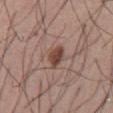Assessment: Recorded during total-body skin imaging; not selected for excision or biopsy. Background: The lesion is on the front of the torso. A male patient aged approximately 55. The tile uses white-light illumination. This image is a 15 mm lesion crop taken from a total-body photograph. About 3 mm across.